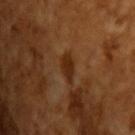Imaged during a routine full-body skin examination; the lesion was not biopsied and no histopathology is available. Approximately 3 mm at its widest. The tile uses cross-polarized illumination. A roughly 15 mm field-of-view crop from a total-body skin photograph. Automated image analysis of the tile measured a lesion–skin lightness drop of about 9 and a normalized border contrast of about 9. It also reported a border-irregularity index near 2.5/10, a within-lesion color-variation index near 1.5/10, and peripheral color asymmetry of about 0.5. A male patient, roughly 65 years of age.